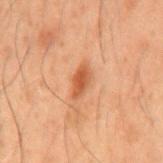Case summary:
– workup: total-body-photography surveillance lesion; no biopsy
– subject: male, approximately 50 years of age
– imaging modality: 15 mm crop, total-body photography
– site: the mid back